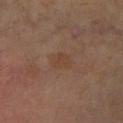Imaged during a routine full-body skin examination; the lesion was not biopsied and no histopathology is available. This image is a 15 mm lesion crop taken from a total-body photograph. Measured at roughly 2.5 mm in maximum diameter. Located on the right lower leg. A male subject, aged around 55. Automated tile analysis of the lesion measured a classifier nevus-likeness of about 0/100 and a lesion-detection confidence of about 100/100. This is a cross-polarized tile.